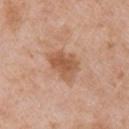biopsy status — no biopsy performed (imaged during a skin exam) | imaging modality — total-body-photography crop, ~15 mm field of view | automated lesion analysis — a mean CIELAB color near L≈56 a*≈22 b*≈34 and a normalized lesion–skin contrast near 7.5; an automated nevus-likeness rating near 50 out of 100 and a detector confidence of about 100 out of 100 that the crop contains a lesion | body site — the right upper arm | subject — female, aged 38–42 | lesion diameter — ≈4 mm.